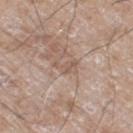The lesion was photographed on a routine skin check and not biopsied; there is no pathology result. On the leg. A 15 mm crop from a total-body photograph taken for skin-cancer surveillance. The subject is a male aged approximately 60. The lesion-visualizer software estimated border irregularity of about 7.5 on a 0–10 scale, a within-lesion color-variation index near 0/10, and peripheral color asymmetry of about 0. And it measured an automated nevus-likeness rating near 0 out of 100 and lesion-presence confidence of about 60/100.